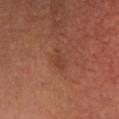Assessment:
Imaged during a routine full-body skin examination; the lesion was not biopsied and no histopathology is available.
Acquisition and patient details:
The tile uses cross-polarized illumination. A 15 mm close-up extracted from a 3D total-body photography capture. From the head or neck. Measured at roughly 3 mm in maximum diameter. The total-body-photography lesion software estimated an area of roughly 3.5 mm², an outline eccentricity of about 0.8 (0 = round, 1 = elongated), and a symmetry-axis asymmetry near 0.25. It also reported a classifier nevus-likeness of about 5/100 and lesion-presence confidence of about 100/100. A female subject roughly 50 years of age.